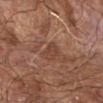The lesion was tiled from a total-body skin photograph and was not biopsied.
A male patient aged 63 to 67.
From the right forearm.
A close-up tile cropped from a whole-body skin photograph, about 15 mm across.